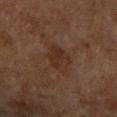| field | value |
|---|---|
| workup | catalogued during a skin exam; not biopsied |
| diameter | ~4 mm (longest diameter) |
| tile lighting | cross-polarized |
| patient | female, roughly 60 years of age |
| TBP lesion metrics | a shape eccentricity near 0.7 |
| anatomic site | the right upper arm |
| imaging modality | total-body-photography crop, ~15 mm field of view |